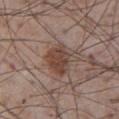biopsy_status: not biopsied; imaged during a skin examination
image:
  source: total-body photography crop
  field_of_view_mm: 15
lighting: white-light
site: front of the torso
lesion_size:
  long_diameter_mm_approx: 4.0
patient:
  sex: male
  age_approx: 75